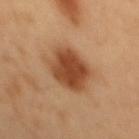biopsy_status: not biopsied; imaged during a skin examination
image:
  source: total-body photography crop
  field_of_view_mm: 15
automated_metrics:
  cielab_L: 46
  cielab_a: 24
  cielab_b: 35
  vs_skin_darker_L: 14.0
  vs_skin_contrast_norm: 10.5
patient:
  sex: male
  age_approx: 50
lighting: cross-polarized
site: mid back
lesion_size:
  long_diameter_mm_approx: 5.0